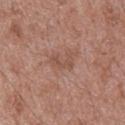<case>
<biopsy_status>not biopsied; imaged during a skin examination</biopsy_status>
<image>
  <source>total-body photography crop</source>
  <field_of_view_mm>15</field_of_view_mm>
</image>
<patient>
  <sex>male</sex>
  <age_approx>50</age_approx>
</patient>
<site>mid back</site>
<lighting>white-light</lighting>
<lesion_size>
  <long_diameter_mm_approx>2.5</long_diameter_mm_approx>
</lesion_size>
</case>